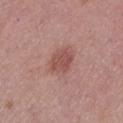No biopsy was performed on this lesion — it was imaged during a full skin examination and was not determined to be concerning.
The patient is a female aged approximately 50.
A region of skin cropped from a whole-body photographic capture, roughly 15 mm wide.
The lesion is located on the leg.